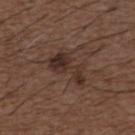Impression:
Imaged during a routine full-body skin examination; the lesion was not biopsied and no histopathology is available.
Acquisition and patient details:
A male patient, aged approximately 50. Automated image analysis of the tile measured a footprint of about 9 mm², a shape eccentricity near 0.9, and a symmetry-axis asymmetry near 0.5. And it measured a border-irregularity index near 7/10, internal color variation of about 4 on a 0–10 scale, and peripheral color asymmetry of about 1.5. It also reported a lesion-detection confidence of about 100/100. The lesion is located on the front of the torso. A close-up tile cropped from a whole-body skin photograph, about 15 mm across.